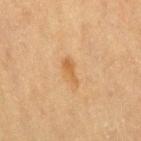workup = no biopsy performed (imaged during a skin exam)
lighting = cross-polarized illumination
location = the right thigh
image source = 15 mm crop, total-body photography
subject = female, about 40 years old
automated metrics = a footprint of about 3 mm², an eccentricity of roughly 0.9, and a symmetry-axis asymmetry near 0.35; an average lesion color of about L≈52 a*≈18 b*≈38 (CIELAB), a lesion–skin lightness drop of about 7, and a normalized lesion–skin contrast near 6.5; a border-irregularity index near 3.5/10, internal color variation of about 0.5 on a 0–10 scale, and a peripheral color-asymmetry measure near 0; a nevus-likeness score of about 10/100 and lesion-presence confidence of about 100/100
lesion diameter = about 3 mm